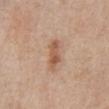Background:
Longest diameter approximately 4.5 mm. This is a white-light tile. A region of skin cropped from a whole-body photographic capture, roughly 15 mm wide. From the abdomen. Automated image analysis of the tile measured an average lesion color of about L≈57 a*≈20 b*≈31 (CIELAB), a lesion–skin lightness drop of about 10, and a normalized lesion–skin contrast near 7. The subject is a male aged approximately 80.